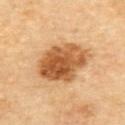Impression:
Captured during whole-body skin photography for melanoma surveillance; the lesion was not biopsied.
Acquisition and patient details:
Located on the upper back. A region of skin cropped from a whole-body photographic capture, roughly 15 mm wide. A male subject aged 83 or older. The tile uses cross-polarized illumination.